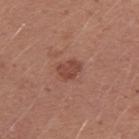Captured during whole-body skin photography for melanoma surveillance; the lesion was not biopsied.
Imaged with white-light lighting.
A male patient aged 23 to 27.
The recorded lesion diameter is about 3 mm.
Cropped from a total-body skin-imaging series; the visible field is about 15 mm.
The lesion is located on the left upper arm.